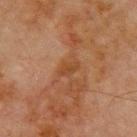Impression: The lesion was photographed on a routine skin check and not biopsied; there is no pathology result. Background: A region of skin cropped from a whole-body photographic capture, roughly 15 mm wide. A male subject, aged 68 to 72. Longest diameter approximately 3 mm. Located on the front of the torso.